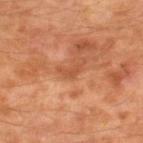The lesion was photographed on a routine skin check and not biopsied; there is no pathology result.
Longest diameter approximately 2.5 mm.
Automated tile analysis of the lesion measured an automated nevus-likeness rating near 0 out of 100 and a detector confidence of about 100 out of 100 that the crop contains a lesion.
The lesion is located on the right lower leg.
The tile uses cross-polarized illumination.
A male subject aged 58–62.
A lesion tile, about 15 mm wide, cut from a 3D total-body photograph.